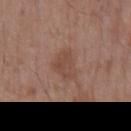Impression: The lesion was tiled from a total-body skin photograph and was not biopsied. Image and clinical context: The total-body-photography lesion software estimated a footprint of about 6.5 mm² and an eccentricity of roughly 0.75. The analysis additionally found an average lesion color of about L≈46 a*≈20 b*≈26 (CIELAB) and a normalized lesion–skin contrast near 5.5. The analysis additionally found a nevus-likeness score of about 10/100 and lesion-presence confidence of about 100/100. The patient is a male roughly 55 years of age. A 15 mm crop from a total-body photograph taken for skin-cancer surveillance. The lesion is located on the mid back. About 3.5 mm across. The tile uses white-light illumination.